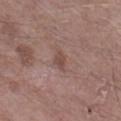The lesion was photographed on a routine skin check and not biopsied; there is no pathology result. Captured under white-light illumination. A region of skin cropped from a whole-body photographic capture, roughly 15 mm wide. About 2.5 mm across. From the right lower leg. The patient is a male about 85 years old or older.